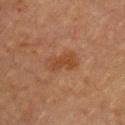follow-up: catalogued during a skin exam; not biopsied | lighting: cross-polarized illumination | patient: female, roughly 60 years of age | TBP lesion metrics: an average lesion color of about L≈40 a*≈21 b*≈32 (CIELAB), roughly 6 lightness units darker than nearby skin, and a lesion-to-skin contrast of about 6.5 (normalized; higher = more distinct); an automated nevus-likeness rating near 15 out of 100 and a detector confidence of about 100 out of 100 that the crop contains a lesion | acquisition: 15 mm crop, total-body photography | body site: the chest | diameter: ≈4 mm.